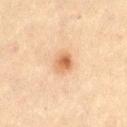  biopsy_status: not biopsied; imaged during a skin examination
  patient:
    sex: female
    age_approx: 45
  lighting: cross-polarized
  lesion_size:
    long_diameter_mm_approx: 2.5
  image:
    source: total-body photography crop
    field_of_view_mm: 15
  site: right thigh